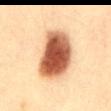Impression:
This lesion was catalogued during total-body skin photography and was not selected for biopsy.
Clinical summary:
A male patient, aged approximately 35. A 15 mm crop from a total-body photograph taken for skin-cancer surveillance. Longest diameter approximately 6.5 mm. Imaged with cross-polarized lighting. The lesion is located on the abdomen.